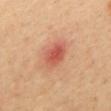Clinical summary: This is a cross-polarized tile. The patient is a male in their 40s. Longest diameter approximately 3.5 mm. This image is a 15 mm lesion crop taken from a total-body photograph. The lesion is on the back.